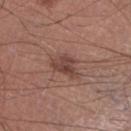The lesion was photographed on a routine skin check and not biopsied; there is no pathology result.
A 15 mm crop from a total-body photograph taken for skin-cancer surveillance.
The lesion is located on the leg.
About 3 mm across.
A male patient aged around 45.
Imaged with white-light lighting.